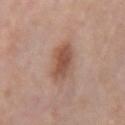notes: imaged on a skin check; not biopsied
site: the right forearm
subject: female, in their mid- to late 60s
TBP lesion metrics: a mean CIELAB color near L≈52 a*≈20 b*≈28, about 10 CIELAB-L* units darker than the surrounding skin, and a normalized lesion–skin contrast near 7.5; a border-irregularity index near 2/10 and a within-lesion color-variation index near 4.5/10; a classifier nevus-likeness of about 85/100
image source: ~15 mm tile from a whole-body skin photo
tile lighting: white-light